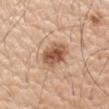Impression: Part of a total-body skin-imaging series; this lesion was reviewed on a skin check and was not flagged for biopsy. Background: A close-up tile cropped from a whole-body skin photograph, about 15 mm across. Located on the left forearm. A male patient, in their 60s. About 4 mm across. This is a white-light tile.